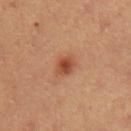Assessment:
Imaged during a routine full-body skin examination; the lesion was not biopsied and no histopathology is available.
Background:
The patient is a female aged approximately 35. A 15 mm close-up extracted from a 3D total-body photography capture. From the left upper arm.